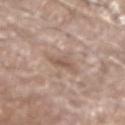follow-up: no biopsy performed (imaged during a skin exam)
location: the left forearm
automated metrics: an area of roughly 3.5 mm², a shape eccentricity near 0.85, and a shape-asymmetry score of about 0.4 (0 = symmetric); an average lesion color of about L≈55 a*≈17 b*≈26 (CIELAB), a lesion–skin lightness drop of about 9, and a lesion-to-skin contrast of about 6.5 (normalized; higher = more distinct); border irregularity of about 4.5 on a 0–10 scale, a color-variation rating of about 1.5/10, and radial color variation of about 0.5
subject: male, aged approximately 65
diameter: ≈3 mm
imaging modality: total-body-photography crop, ~15 mm field of view
illumination: white-light